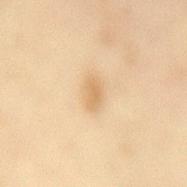Q: Was a biopsy performed?
A: no biopsy performed (imaged during a skin exam)
Q: Where on the body is the lesion?
A: the back
Q: Who is the patient?
A: female, aged 58 to 62
Q: What is the imaging modality?
A: ~15 mm crop, total-body skin-cancer survey